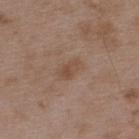{"biopsy_status": "not biopsied; imaged during a skin examination", "patient": {"sex": "male", "age_approx": 50}, "image": {"source": "total-body photography crop", "field_of_view_mm": 15}, "site": "back", "lesion_size": {"long_diameter_mm_approx": 3.0}, "automated_metrics": {"cielab_L": 48, "cielab_a": 17, "cielab_b": 27, "vs_skin_darker_L": 6.0, "vs_skin_contrast_norm": 5.5, "border_irregularity_0_10": 2.0, "color_variation_0_10": 3.0, "peripheral_color_asymmetry": 1.5, "nevus_likeness_0_100": 0, "lesion_detection_confidence_0_100": 100}, "lighting": "white-light"}